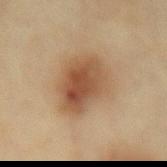  lighting: cross-polarized
  image:
    source: total-body photography crop
    field_of_view_mm: 15
  site: lower back
  lesion_size:
    long_diameter_mm_approx: 6.0
  automated_metrics:
    cielab_L: 48
    cielab_a: 17
    cielab_b: 31
    vs_skin_darker_L: 11.0
    vs_skin_contrast_norm: 8.5
    peripheral_color_asymmetry: 1.5
    nevus_likeness_0_100: 100
  patient:
    sex: female
    age_approx: 60